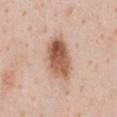Assessment:
No biopsy was performed on this lesion — it was imaged during a full skin examination and was not determined to be concerning.
Acquisition and patient details:
Cropped from a whole-body photographic skin survey; the tile spans about 15 mm. Longest diameter approximately 5.5 mm. The lesion is on the chest. The patient is a female in their mid-40s. This is a white-light tile.